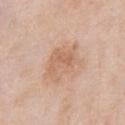<lesion>
<site>chest</site>
<image>
  <source>total-body photography crop</source>
  <field_of_view_mm>15</field_of_view_mm>
</image>
<patient>
  <sex>male</sex>
  <age_approx>55</age_approx>
</patient>
<lighting>white-light</lighting>
</lesion>